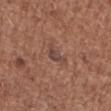Notes:
* patient · female, aged approximately 50
* imaging modality · ~15 mm crop, total-body skin-cancer survey
* body site · the arm